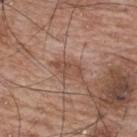Assessment:
The lesion was photographed on a routine skin check and not biopsied; there is no pathology result.
Acquisition and patient details:
Imaged with white-light lighting. A roughly 15 mm field-of-view crop from a total-body skin photograph. A male patient in their 70s. On the upper back. Measured at roughly 3.5 mm in maximum diameter.